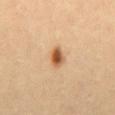No biopsy was performed on this lesion — it was imaged during a full skin examination and was not determined to be concerning. The patient is a female aged 33–37. A close-up tile cropped from a whole-body skin photograph, about 15 mm across. Imaged with cross-polarized lighting. The lesion is located on the abdomen. The lesion's longest dimension is about 2.5 mm. The total-body-photography lesion software estimated an area of roughly 4.5 mm², an outline eccentricity of about 0.7 (0 = round, 1 = elongated), and a shape-asymmetry score of about 0.2 (0 = symmetric). The analysis additionally found an average lesion color of about L≈56 a*≈23 b*≈38 (CIELAB), roughly 15 lightness units darker than nearby skin, and a normalized lesion–skin contrast near 10. The software also gave border irregularity of about 1.5 on a 0–10 scale, a within-lesion color-variation index near 6/10, and a peripheral color-asymmetry measure near 2. And it measured a classifier nevus-likeness of about 100/100 and a lesion-detection confidence of about 100/100.